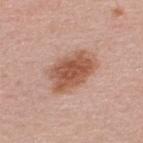Q: Is there a histopathology result?
A: imaged on a skin check; not biopsied